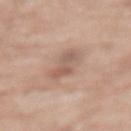Clinical summary:
On the mid back. The patient is a female aged 73–77. An algorithmic analysis of the crop reported a lesion area of about 5 mm² and a symmetry-axis asymmetry near 0.35. The software also gave a mean CIELAB color near L≈57 a*≈18 b*≈26 and a lesion–skin lightness drop of about 9. The analysis additionally found a lesion-detection confidence of about 100/100. Longest diameter approximately 4 mm. Captured under white-light illumination. Cropped from a total-body skin-imaging series; the visible field is about 15 mm.